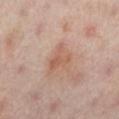Case summary:
* patient · female, in their 40s
* body site · the right leg
* lighting · cross-polarized
* imaging modality · 15 mm crop, total-body photography